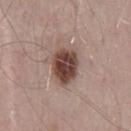{
  "biopsy_status": "not biopsied; imaged during a skin examination",
  "site": "mid back",
  "patient": {
    "sex": "male",
    "age_approx": 70
  },
  "image": {
    "source": "total-body photography crop",
    "field_of_view_mm": 15
  },
  "automated_metrics": {
    "cielab_L": 43,
    "cielab_a": 19,
    "cielab_b": 23,
    "vs_skin_darker_L": 17.0,
    "vs_skin_contrast_norm": 12.5,
    "nevus_likeness_0_100": 95,
    "lesion_detection_confidence_0_100": 100
  },
  "lighting": "white-light"
}